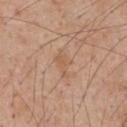| feature | finding |
|---|---|
| workup | total-body-photography surveillance lesion; no biopsy |
| lesion size | ≈3 mm |
| subject | male, aged approximately 60 |
| body site | the chest |
| image source | ~15 mm tile from a whole-body skin photo |
| lighting | white-light |